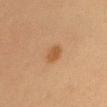The lesion was photographed on a routine skin check and not biopsied; there is no pathology result.
The lesion-visualizer software estimated an area of roughly 4 mm², an outline eccentricity of about 0.6 (0 = round, 1 = elongated), and two-axis asymmetry of about 0.25. And it measured a nevus-likeness score of about 90/100 and lesion-presence confidence of about 100/100.
From the head or neck.
This image is a 15 mm lesion crop taken from a total-body photograph.
Captured under cross-polarized illumination.
A female patient aged 28 to 32.